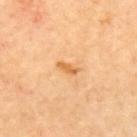This lesion was catalogued during total-body skin photography and was not selected for biopsy.
From the upper back.
About 2.5 mm across.
A lesion tile, about 15 mm wide, cut from a 3D total-body photograph.
The patient is a male in their mid- to late 60s.
The tile uses cross-polarized illumination.
An algorithmic analysis of the crop reported a footprint of about 2.5 mm², an eccentricity of roughly 0.9, and a symmetry-axis asymmetry near 0.3. The analysis additionally found a mean CIELAB color near L≈67 a*≈25 b*≈47, a lesion–skin lightness drop of about 10, and a normalized lesion–skin contrast near 7.5. The software also gave a nevus-likeness score of about 5/100 and lesion-presence confidence of about 100/100.